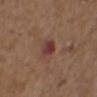Recorded during total-body skin imaging; not selected for excision or biopsy.
Captured under white-light illumination.
A male subject, aged 73–77.
A roughly 15 mm field-of-view crop from a total-body skin photograph.
Automated image analysis of the tile measured a footprint of about 4.5 mm² and two-axis asymmetry of about 0.2. It also reported a mean CIELAB color near L≈37 a*≈24 b*≈22, about 10 CIELAB-L* units darker than the surrounding skin, and a normalized lesion–skin contrast near 9.5. It also reported border irregularity of about 2 on a 0–10 scale, a within-lesion color-variation index near 5.5/10, and radial color variation of about 2.
The lesion is on the chest.
The recorded lesion diameter is about 2.5 mm.